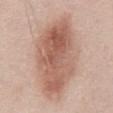Part of a total-body skin-imaging series; this lesion was reviewed on a skin check and was not flagged for biopsy.
The lesion's longest dimension is about 10 mm.
The tile uses white-light illumination.
The lesion-visualizer software estimated a mean CIELAB color near L≈59 a*≈21 b*≈28, roughly 13 lightness units darker than nearby skin, and a normalized border contrast of about 8.
Cropped from a total-body skin-imaging series; the visible field is about 15 mm.
A male patient, roughly 50 years of age.
Located on the abdomen.